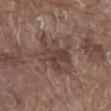Imaged during a routine full-body skin examination; the lesion was not biopsied and no histopathology is available. A male patient, aged 78 to 82. Cropped from a total-body skin-imaging series; the visible field is about 15 mm. The lesion is on the abdomen.